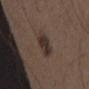<lesion>
  <biopsy_status>not biopsied; imaged during a skin examination</biopsy_status>
  <site>left upper arm</site>
  <patient>
    <sex>male</sex>
    <age_approx>50</age_approx>
  </patient>
  <image>
    <source>total-body photography crop</source>
    <field_of_view_mm>15</field_of_view_mm>
  </image>
  <lesion_size>
    <long_diameter_mm_approx>4.0</long_diameter_mm_approx>
  </lesion_size>
  <lighting>white-light</lighting>
</lesion>